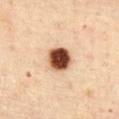Clinical impression:
Part of a total-body skin-imaging series; this lesion was reviewed on a skin check and was not flagged for biopsy.
Clinical summary:
Automated tile analysis of the lesion measured a lesion color around L≈47 a*≈24 b*≈32 in CIELAB and a normalized border contrast of about 17.5. The software also gave a border-irregularity rating of about 1/10, a color-variation rating of about 6/10, and radial color variation of about 1.5. A female patient, in their mid-40s. Located on the chest. A 15 mm close-up tile from a total-body photography series done for melanoma screening. About 3 mm across.